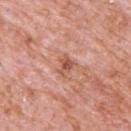The lesion was tiled from a total-body skin photograph and was not biopsied.
A close-up tile cropped from a whole-body skin photograph, about 15 mm across.
A male subject, aged 78–82.
The lesion is located on the back.